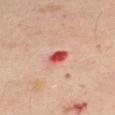notes: catalogued during a skin exam; not biopsied | imaging modality: total-body-photography crop, ~15 mm field of view | TBP lesion metrics: a footprint of about 3.5 mm², an outline eccentricity of about 0.75 (0 = round, 1 = elongated), and a shape-asymmetry score of about 0.25 (0 = symmetric); border irregularity of about 2 on a 0–10 scale, internal color variation of about 3.5 on a 0–10 scale, and peripheral color asymmetry of about 1; a classifier nevus-likeness of about 0/100 | location: the right leg | lesion size: ~2.5 mm (longest diameter) | patient: female, roughly 40 years of age.